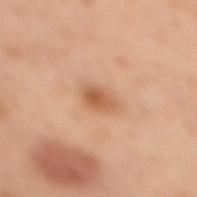notes: no biopsy performed (imaged during a skin exam) | lesion size: ~2.5 mm (longest diameter) | subject: female, aged 53–57 | illumination: cross-polarized | image-analysis metrics: a footprint of about 3.5 mm², an outline eccentricity of about 0.75 (0 = round, 1 = elongated), and a shape-asymmetry score of about 0.2 (0 = symmetric); an average lesion color of about L≈47 a*≈20 b*≈30 (CIELAB) and a normalized border contrast of about 7.5; a nevus-likeness score of about 85/100 and a detector confidence of about 100 out of 100 that the crop contains a lesion | body site: the upper back | image: ~15 mm tile from a whole-body skin photo.